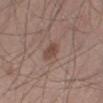<record>
<biopsy_status>not biopsied; imaged during a skin examination</biopsy_status>
<image>
  <source>total-body photography crop</source>
  <field_of_view_mm>15</field_of_view_mm>
</image>
<patient>
  <sex>male</sex>
  <age_approx>60</age_approx>
</patient>
<lighting>white-light</lighting>
<automated_metrics>
  <border_irregularity_0_10>1.5</border_irregularity_0_10>
  <color_variation_0_10>2.0</color_variation_0_10>
  <nevus_likeness_0_100>65</nevus_likeness_0_100>
  <lesion_detection_confidence_0_100>100</lesion_detection_confidence_0_100>
</automated_metrics>
<site>right thigh</site>
</record>